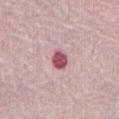| field | value |
|---|---|
| biopsy status | total-body-photography surveillance lesion; no biopsy |
| diameter | ≈3 mm |
| patient | female, in their mid- to late 60s |
| image source | 15 mm crop, total-body photography |
| automated metrics | a mean CIELAB color near L≈53 a*≈31 b*≈16, about 17 CIELAB-L* units darker than the surrounding skin, and a normalized lesion–skin contrast near 11; a border-irregularity rating of about 2/10, internal color variation of about 2 on a 0–10 scale, and radial color variation of about 0.5 |
| lighting | white-light illumination |
| location | the abdomen |